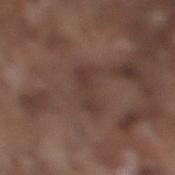{
  "biopsy_status": "not biopsied; imaged during a skin examination",
  "automated_metrics": {
    "vs_skin_darker_L": 6.0,
    "vs_skin_contrast_norm": 5.5
  },
  "site": "right lower leg",
  "lesion_size": {
    "long_diameter_mm_approx": 4.0
  },
  "lighting": "white-light",
  "patient": {
    "sex": "male",
    "age_approx": 75
  },
  "image": {
    "source": "total-body photography crop",
    "field_of_view_mm": 15
  }
}